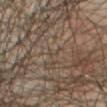This lesion was catalogued during total-body skin photography and was not selected for biopsy. The tile uses cross-polarized illumination. This image is a 15 mm lesion crop taken from a total-body photograph. Measured at roughly 1.5 mm in maximum diameter. The lesion is located on the abdomen. The patient is a male aged 63 to 67.